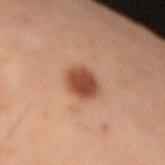Impression:
Captured during whole-body skin photography for melanoma surveillance; the lesion was not biopsied.
Context:
Longest diameter approximately 3 mm. Captured under cross-polarized illumination. A male patient, approximately 70 years of age. This image is a 15 mm lesion crop taken from a total-body photograph. From the left forearm.